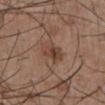Q: Was a biopsy performed?
A: imaged on a skin check; not biopsied
Q: Patient demographics?
A: male, aged approximately 55
Q: How was the tile lit?
A: white-light illumination
Q: Lesion location?
A: the abdomen
Q: How large is the lesion?
A: ≈3.5 mm
Q: What did automated image analysis measure?
A: an area of roughly 6.5 mm², a shape eccentricity near 0.7, and a shape-asymmetry score of about 0.3 (0 = symmetric); an average lesion color of about L≈42 a*≈19 b*≈26 (CIELAB), a lesion–skin lightness drop of about 9, and a lesion-to-skin contrast of about 7.5 (normalized; higher = more distinct); a nevus-likeness score of about 70/100 and lesion-presence confidence of about 100/100
Q: What kind of image is this?
A: ~15 mm tile from a whole-body skin photo